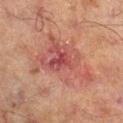Recorded during total-body skin imaging; not selected for excision or biopsy. A roughly 15 mm field-of-view crop from a total-body skin photograph. A male patient aged approximately 65. From the left lower leg.